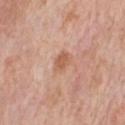Clinical impression:
No biopsy was performed on this lesion — it was imaged during a full skin examination and was not determined to be concerning.
Clinical summary:
A male patient aged 58 to 62. On the chest. A close-up tile cropped from a whole-body skin photograph, about 15 mm across.